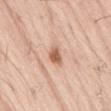| field | value |
|---|---|
| lighting | white-light illumination |
| TBP lesion metrics | a nevus-likeness score of about 75/100 and a detector confidence of about 100 out of 100 that the crop contains a lesion |
| subject | male, roughly 55 years of age |
| location | the lower back |
| image source | 15 mm crop, total-body photography |